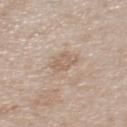Context: The lesion is on the upper back. Captured under white-light illumination. A close-up tile cropped from a whole-body skin photograph, about 15 mm across. A female patient aged around 30.